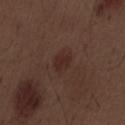Case summary:
• notes · catalogued during a skin exam; not biopsied
• anatomic site · the abdomen
• tile lighting · white-light
• subject · male, aged around 70
• acquisition · total-body-photography crop, ~15 mm field of view
• lesion diameter · ≈2.5 mm